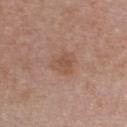| feature | finding |
|---|---|
| workup | no biopsy performed (imaged during a skin exam) |
| lesion size | ≈3 mm |
| anatomic site | the chest |
| patient | female, about 40 years old |
| lighting | white-light |
| image | total-body-photography crop, ~15 mm field of view |